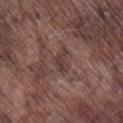  patient:
    sex: male
    age_approx: 75
  lighting: white-light
  site: leg
  image:
    source: total-body photography crop
    field_of_view_mm: 15